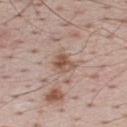A male patient, in their 70s. The tile uses white-light illumination. On the upper back. The lesion-visualizer software estimated an area of roughly 5 mm² and a symmetry-axis asymmetry near 0.3. And it measured an average lesion color of about L≈54 a*≈18 b*≈27 (CIELAB), roughly 10 lightness units darker than nearby skin, and a normalized border contrast of about 7.5. The analysis additionally found a lesion-detection confidence of about 100/100. Cropped from a whole-body photographic skin survey; the tile spans about 15 mm. About 3 mm across.